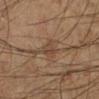The lesion was photographed on a routine skin check and not biopsied; there is no pathology result. On the leg. Cropped from a whole-body photographic skin survey; the tile spans about 15 mm. A male patient, roughly 60 years of age.